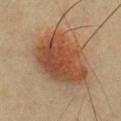This lesion was catalogued during total-body skin photography and was not selected for biopsy. The total-body-photography lesion software estimated an eccentricity of roughly 0.65. It also reported a lesion–skin lightness drop of about 11 and a lesion-to-skin contrast of about 9 (normalized; higher = more distinct). And it measured a classifier nevus-likeness of about 100/100 and a lesion-detection confidence of about 100/100. A 15 mm close-up extracted from a 3D total-body photography capture. On the chest. The recorded lesion diameter is about 7.5 mm. The subject is a male aged approximately 40.